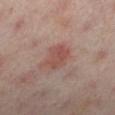Clinical impression:
Part of a total-body skin-imaging series; this lesion was reviewed on a skin check and was not flagged for biopsy.
Acquisition and patient details:
The total-body-photography lesion software estimated a lesion color around L≈51 a*≈23 b*≈24 in CIELAB, about 8 CIELAB-L* units darker than the surrounding skin, and a normalized lesion–skin contrast near 6. The analysis additionally found a nevus-likeness score of about 35/100 and lesion-presence confidence of about 100/100. The lesion is located on the right leg. The recorded lesion diameter is about 3.5 mm. A female patient, aged around 40. A 15 mm crop from a total-body photograph taken for skin-cancer surveillance.